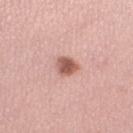Part of a total-body skin-imaging series; this lesion was reviewed on a skin check and was not flagged for biopsy. Longest diameter approximately 2.5 mm. A lesion tile, about 15 mm wide, cut from a 3D total-body photograph. Located on the left lower leg. Imaged with white-light lighting. A female subject approximately 35 years of age.